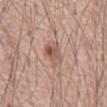{"biopsy_status": "not biopsied; imaged during a skin examination", "image": {"source": "total-body photography crop", "field_of_view_mm": 15}, "patient": {"sex": "male", "age_approx": 70}, "lighting": "white-light", "site": "front of the torso", "lesion_size": {"long_diameter_mm_approx": 3.5}}